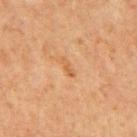- follow-up — no biopsy performed (imaged during a skin exam)
- subject — male, aged around 65
- imaging modality — total-body-photography crop, ~15 mm field of view
- diameter — ~2.5 mm (longest diameter)
- lighting — cross-polarized illumination
- anatomic site — the mid back